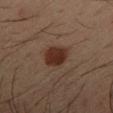- workup — total-body-photography surveillance lesion; no biopsy
- body site — the arm
- lesion size — about 3.5 mm
- lighting — cross-polarized
- imaging modality — ~15 mm crop, total-body skin-cancer survey
- subject — male, in their 40s